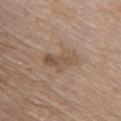This lesion was catalogued during total-body skin photography and was not selected for biopsy. A female patient aged 63–67. The lesion is on the upper back. A lesion tile, about 15 mm wide, cut from a 3D total-body photograph. Measured at roughly 5 mm in maximum diameter.